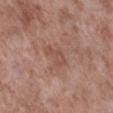| feature | finding |
|---|---|
| workup | total-body-photography surveillance lesion; no biopsy |
| body site | the leg |
| subject | male, in their mid-50s |
| size | ≈3 mm |
| acquisition | ~15 mm crop, total-body skin-cancer survey |
| tile lighting | white-light |